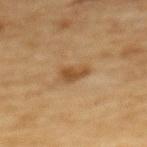Assessment:
This lesion was catalogued during total-body skin photography and was not selected for biopsy.
Context:
Located on the upper back. Automated image analysis of the tile measured a lesion color around L≈42 a*≈17 b*≈33 in CIELAB and roughly 9 lightness units darker than nearby skin. And it measured a classifier nevus-likeness of about 70/100. About 3 mm across. A female patient, roughly 55 years of age. A region of skin cropped from a whole-body photographic capture, roughly 15 mm wide.